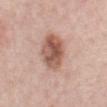Assessment: No biopsy was performed on this lesion — it was imaged during a full skin examination and was not determined to be concerning. Image and clinical context: This is a white-light tile. On the abdomen. A close-up tile cropped from a whole-body skin photograph, about 15 mm across. The subject is a female in their mid-60s.